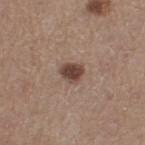{"biopsy_status": "not biopsied; imaged during a skin examination", "image": {"source": "total-body photography crop", "field_of_view_mm": 15}, "automated_metrics": {"cielab_L": 43, "cielab_a": 17, "cielab_b": 23, "vs_skin_darker_L": 14.0, "vs_skin_contrast_norm": 10.5, "lesion_detection_confidence_0_100": 100}, "lesion_size": {"long_diameter_mm_approx": 2.5}, "lighting": "white-light", "patient": {"sex": "female", "age_approx": 45}, "site": "left thigh"}